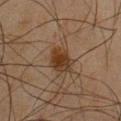The subject is a male aged 43 to 47. Cropped from a whole-body photographic skin survey; the tile spans about 15 mm. Located on the chest.